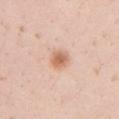{
  "biopsy_status": "not biopsied; imaged during a skin examination",
  "image": {
    "source": "total-body photography crop",
    "field_of_view_mm": 15
  },
  "patient": {
    "sex": "female",
    "age_approx": 20
  },
  "site": "left upper arm",
  "lighting": "white-light",
  "automated_metrics": {
    "area_mm2_approx": 5.0,
    "border_irregularity_0_10": 1.5,
    "color_variation_0_10": 4.0,
    "peripheral_color_asymmetry": 1.0,
    "lesion_detection_confidence_0_100": 100
  }
}